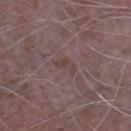Part of a total-body skin-imaging series; this lesion was reviewed on a skin check and was not flagged for biopsy.
A male subject aged around 65.
A 15 mm close-up extracted from a 3D total-body photography capture.
Longest diameter approximately 3 mm.
The lesion-visualizer software estimated an automated nevus-likeness rating near 0 out of 100.
The lesion is on the chest.
This is a white-light tile.